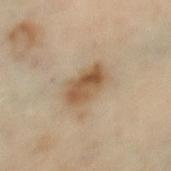Impression: The lesion was photographed on a routine skin check and not biopsied; there is no pathology result. Acquisition and patient details: Automated tile analysis of the lesion measured an area of roughly 10 mm², an eccentricity of roughly 0.85, and a symmetry-axis asymmetry near 0.15. The analysis additionally found an average lesion color of about L≈54 a*≈15 b*≈33 (CIELAB) and a lesion-to-skin contrast of about 8.5 (normalized; higher = more distinct). It also reported an automated nevus-likeness rating near 45 out of 100 and a detector confidence of about 100 out of 100 that the crop contains a lesion. A close-up tile cropped from a whole-body skin photograph, about 15 mm across. Located on the left thigh. About 4.5 mm across. A female patient, aged around 60. Imaged with cross-polarized lighting.